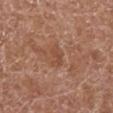Imaged during a routine full-body skin examination; the lesion was not biopsied and no histopathology is available. Measured at roughly 2.5 mm in maximum diameter. The subject is a male about 75 years old. A 15 mm crop from a total-body photograph taken for skin-cancer surveillance. The lesion is located on the right lower leg.